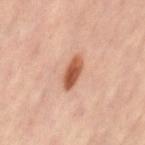Case summary:
* workup: no biopsy performed (imaged during a skin exam)
* diameter: about 4 mm
* acquisition: total-body-photography crop, ~15 mm field of view
* site: the lower back
* subject: female, in their 40s
* illumination: cross-polarized illumination